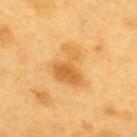Imaged during a routine full-body skin examination; the lesion was not biopsied and no histopathology is available.